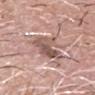workup = catalogued during a skin exam; not biopsied | imaging modality = total-body-photography crop, ~15 mm field of view | illumination = white-light | location = the head or neck | TBP lesion metrics = a shape eccentricity near 0.75 and a symmetry-axis asymmetry near 0.35; a lesion–skin lightness drop of about 11; a border-irregularity rating of about 4.5/10, a color-variation rating of about 6/10, and a peripheral color-asymmetry measure near 2; a classifier nevus-likeness of about 0/100 and a lesion-detection confidence of about 80/100 | diameter = ~4.5 mm (longest diameter) | subject = male, aged around 70.